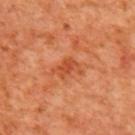The lesion was photographed on a routine skin check and not biopsied; there is no pathology result.
A 15 mm crop from a total-body photograph taken for skin-cancer surveillance.
The lesion is on the upper back.
A female patient, aged approximately 40.